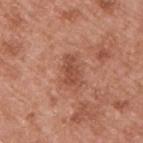The subject is a male aged 53 to 57. This image is a 15 mm lesion crop taken from a total-body photograph. The lesion is on the upper back. Captured under white-light illumination. About 3.5 mm across.